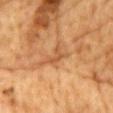The lesion was photographed on a routine skin check and not biopsied; there is no pathology result. The lesion is located on the front of the torso. A 15 mm close-up extracted from a 3D total-body photography capture. The lesion-visualizer software estimated a lesion area of about 3 mm², a shape eccentricity near 0.8, and a shape-asymmetry score of about 0.55 (0 = symmetric). And it measured a classifier nevus-likeness of about 0/100 and lesion-presence confidence of about 95/100. A male patient aged 83 or older. This is a cross-polarized tile. Longest diameter approximately 2.5 mm.